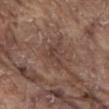{"biopsy_status": "not biopsied; imaged during a skin examination", "lesion_size": {"long_diameter_mm_approx": 3.5}, "image": {"source": "total-body photography crop", "field_of_view_mm": 15}, "lighting": "white-light", "site": "abdomen", "patient": {"sex": "male", "age_approx": 80}}